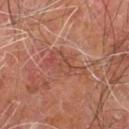No biopsy was performed on this lesion — it was imaged during a full skin examination and was not determined to be concerning. This image is a 15 mm lesion crop taken from a total-body photograph. The lesion-visualizer software estimated an area of roughly 10 mm², an outline eccentricity of about 0.9 (0 = round, 1 = elongated), and a shape-asymmetry score of about 0.4 (0 = symmetric). The software also gave an average lesion color of about L≈46 a*≈24 b*≈28 (CIELAB), a lesion–skin lightness drop of about 6, and a lesion-to-skin contrast of about 5 (normalized; higher = more distinct). A male patient, aged around 60. About 5.5 mm across. Located on the right leg. Captured under cross-polarized illumination.